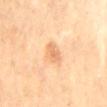lesion diameter = about 3 mm | lighting = cross-polarized | subject = female, aged 68 to 72 | body site = the mid back | image source = ~15 mm crop, total-body skin-cancer survey.